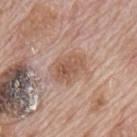Part of a total-body skin-imaging series; this lesion was reviewed on a skin check and was not flagged for biopsy. A roughly 15 mm field-of-view crop from a total-body skin photograph. Measured at roughly 4 mm in maximum diameter. The lesion-visualizer software estimated an average lesion color of about L≈55 a*≈20 b*≈28 (CIELAB), a lesion–skin lightness drop of about 9, and a normalized border contrast of about 6.5. The analysis additionally found an automated nevus-likeness rating near 25 out of 100 and a lesion-detection confidence of about 100/100. A male subject, about 70 years old. The tile uses white-light illumination. The lesion is located on the mid back.